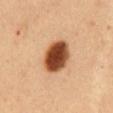Impression: The lesion was tiled from a total-body skin photograph and was not biopsied. Acquisition and patient details: The lesion is located on the abdomen. An algorithmic analysis of the crop reported a lesion area of about 14 mm², an eccentricity of roughly 0.65, and two-axis asymmetry of about 0.1. The analysis additionally found an average lesion color of about L≈48 a*≈25 b*≈37 (CIELAB) and a normalized border contrast of about 15. The analysis additionally found a border-irregularity index near 1/10, internal color variation of about 6.5 on a 0–10 scale, and radial color variation of about 1.5. A close-up tile cropped from a whole-body skin photograph, about 15 mm across. A male subject, about 50 years old. Imaged with cross-polarized lighting.